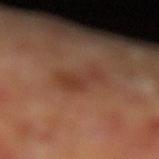This lesion was catalogued during total-body skin photography and was not selected for biopsy. A 15 mm close-up extracted from a 3D total-body photography capture. A male subject, aged 58–62. Imaged with cross-polarized lighting. On the left lower leg.